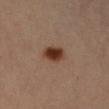Clinical impression: The lesion was photographed on a routine skin check and not biopsied; there is no pathology result. Clinical summary: From the right forearm. A 15 mm close-up tile from a total-body photography series done for melanoma screening. The total-body-photography lesion software estimated a lesion area of about 6 mm² and an eccentricity of roughly 0.65. The analysis additionally found a mean CIELAB color near L≈34 a*≈20 b*≈27, a lesion–skin lightness drop of about 14, and a normalized lesion–skin contrast near 12. Imaged with cross-polarized lighting. Approximately 3 mm at its widest. The subject is a female approximately 45 years of age.